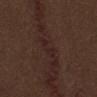follow-up = catalogued during a skin exam; not biopsied | image = total-body-photography crop, ~15 mm field of view | patient = male, in their 70s | size = ~3 mm (longest diameter) | site = the mid back | automated lesion analysis = a lesion area of about 3.5 mm², a shape eccentricity near 0.9, and two-axis asymmetry of about 0.45; about 4 CIELAB-L* units darker than the surrounding skin and a normalized border contrast of about 6; a border-irregularity rating of about 5.5/10 and a color-variation rating of about 0/10; an automated nevus-likeness rating near 0 out of 100.